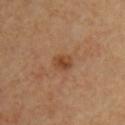The lesion was photographed on a routine skin check and not biopsied; there is no pathology result. Measured at roughly 2.5 mm in maximum diameter. On the chest. A male subject, aged around 55. This image is a 15 mm lesion crop taken from a total-body photograph. Captured under cross-polarized illumination.